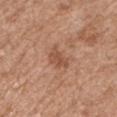Imaged during a routine full-body skin examination; the lesion was not biopsied and no histopathology is available.
The patient is a male about 55 years old.
A lesion tile, about 15 mm wide, cut from a 3D total-body photograph.
Captured under white-light illumination.
From the right upper arm.
The recorded lesion diameter is about 3 mm.
The total-body-photography lesion software estimated an average lesion color of about L≈51 a*≈22 b*≈32 (CIELAB), roughly 9 lightness units darker than nearby skin, and a normalized border contrast of about 6.5.